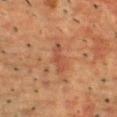biopsy status: total-body-photography surveillance lesion; no biopsy | patient: male, in their mid- to late 50s | acquisition: 15 mm crop, total-body photography | anatomic site: the upper back | tile lighting: cross-polarized illumination.